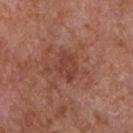notes: imaged on a skin check; not biopsied | image-analysis metrics: a mean CIELAB color near L≈42 a*≈24 b*≈27, a lesion–skin lightness drop of about 7, and a normalized border contrast of about 5.5; a nevus-likeness score of about 0/100 and a detector confidence of about 100 out of 100 that the crop contains a lesion | subject: male, roughly 65 years of age | size: about 3.5 mm | image: total-body-photography crop, ~15 mm field of view | tile lighting: white-light illumination | site: the chest.